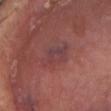Captured during whole-body skin photography for melanoma surveillance; the lesion was not biopsied. An algorithmic analysis of the crop reported an area of roughly 7 mm² and a shape eccentricity near 0.85. And it measured border irregularity of about 4 on a 0–10 scale, internal color variation of about 4.5 on a 0–10 scale, and radial color variation of about 1.5. The software also gave a nevus-likeness score of about 0/100. A 15 mm close-up tile from a total-body photography series done for melanoma screening. The patient is a male roughly 80 years of age. The lesion's longest dimension is about 4 mm. Imaged with white-light lighting. On the head or neck.